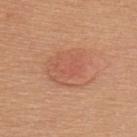Notes:
* workup · total-body-photography surveillance lesion; no biopsy
* location · the upper back
* patient · female, aged approximately 60
* illumination · white-light illumination
* acquisition · ~15 mm tile from a whole-body skin photo